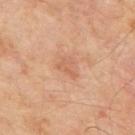Imaged during a routine full-body skin examination; the lesion was not biopsied and no histopathology is available. The lesion is on the back. A male subject in their 70s. Approximately 2.5 mm at its widest. A 15 mm close-up tile from a total-body photography series done for melanoma screening. This is a cross-polarized tile. Automated image analysis of the tile measured an average lesion color of about L≈59 a*≈23 b*≈34 (CIELAB), a lesion–skin lightness drop of about 7, and a normalized lesion–skin contrast near 4.5. The software also gave border irregularity of about 6 on a 0–10 scale, internal color variation of about 1 on a 0–10 scale, and radial color variation of about 0.5.